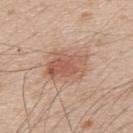Acquisition and patient details:
The tile uses white-light illumination. A male patient aged 48–52. Automated image analysis of the tile measured an area of roughly 14 mm², a shape eccentricity near 0.7, and a shape-asymmetry score of about 0.2 (0 = symmetric). The software also gave a border-irregularity rating of about 2.5/10 and radial color variation of about 1.5. From the upper back. The recorded lesion diameter is about 5 mm. A lesion tile, about 15 mm wide, cut from a 3D total-body photograph.